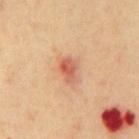The tile uses cross-polarized illumination.
On the abdomen.
A roughly 15 mm field-of-view crop from a total-body skin photograph.
Automated tile analysis of the lesion measured a lesion area of about 6 mm², an outline eccentricity of about 0.7 (0 = round, 1 = elongated), and a shape-asymmetry score of about 0.25 (0 = symmetric). It also reported roughly 11 lightness units darker than nearby skin and a lesion-to-skin contrast of about 7 (normalized; higher = more distinct). And it measured a classifier nevus-likeness of about 0/100 and a lesion-detection confidence of about 100/100.
The patient is a male about 70 years old.